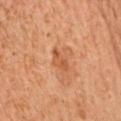Q: Was this lesion biopsied?
A: imaged on a skin check; not biopsied
Q: How was the tile lit?
A: cross-polarized illumination
Q: What is the anatomic site?
A: the chest
Q: How large is the lesion?
A: ≈3 mm
Q: What kind of image is this?
A: total-body-photography crop, ~15 mm field of view
Q: Patient demographics?
A: male, approximately 60 years of age